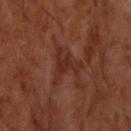notes: imaged on a skin check; not biopsied
lesion diameter: ~4.5 mm (longest diameter)
image-analysis metrics: an area of roughly 7 mm², an outline eccentricity of about 0.6 (0 = round, 1 = elongated), and a shape-asymmetry score of about 0.65 (0 = symmetric); border irregularity of about 9.5 on a 0–10 scale, a color-variation rating of about 1.5/10, and radial color variation of about 0.5
subject: male, aged 58 to 62
location: the head or neck
image source: 15 mm crop, total-body photography